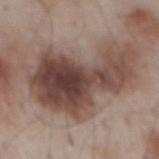biopsy_status: not biopsied; imaged during a skin examination
lesion_size:
  long_diameter_mm_approx: 11.0
automated_metrics:
  eccentricity: 0.85
  shape_asymmetry: 0.5
  nevus_likeness_0_100: 0
lighting: white-light
image:
  source: total-body photography crop
  field_of_view_mm: 15
site: mid back
patient:
  sex: male
  age_approx: 55